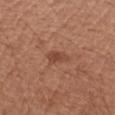Part of a total-body skin-imaging series; this lesion was reviewed on a skin check and was not flagged for biopsy. A 15 mm crop from a total-body photograph taken for skin-cancer surveillance. Imaged with white-light lighting. The patient is a female aged around 40. The lesion is on the right upper arm. Measured at roughly 2.5 mm in maximum diameter.